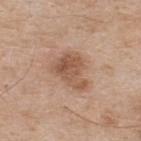Assessment:
Captured during whole-body skin photography for melanoma surveillance; the lesion was not biopsied.
Context:
On the upper back. Captured under white-light illumination. Approximately 4.5 mm at its widest. Automated tile analysis of the lesion measured a footprint of about 12 mm², an eccentricity of roughly 0.7, and a symmetry-axis asymmetry near 0.4. The software also gave a lesion color around L≈54 a*≈19 b*≈30 in CIELAB. The analysis additionally found a within-lesion color-variation index near 4/10 and radial color variation of about 1.5. It also reported a classifier nevus-likeness of about 30/100 and lesion-presence confidence of about 100/100. A 15 mm close-up tile from a total-body photography series done for melanoma screening. A male patient, about 75 years old.